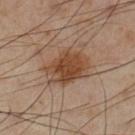<case>
<biopsy_status>not biopsied; imaged during a skin examination</biopsy_status>
<image>
  <source>total-body photography crop</source>
  <field_of_view_mm>15</field_of_view_mm>
</image>
<site>left lower leg</site>
<automated_metrics>
  <nevus_likeness_0_100>90</nevus_likeness_0_100>
  <lesion_detection_confidence_0_100>100</lesion_detection_confidence_0_100>
</automated_metrics>
<patient>
  <sex>male</sex>
  <age_approx>55</age_approx>
</patient>
</case>